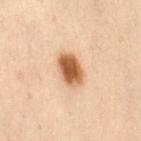Notes:
• notes · imaged on a skin check; not biopsied
• imaging modality · ~15 mm crop, total-body skin-cancer survey
• size · ~4 mm (longest diameter)
• illumination · cross-polarized
• subject · female, roughly 55 years of age
• anatomic site · the abdomen